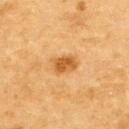{
  "biopsy_status": "not biopsied; imaged during a skin examination",
  "image": {
    "source": "total-body photography crop",
    "field_of_view_mm": 15
  },
  "lighting": "cross-polarized",
  "patient": {
    "sex": "male",
    "age_approx": "85+"
  },
  "site": "upper back",
  "lesion_size": {
    "long_diameter_mm_approx": 3.0
  }
}